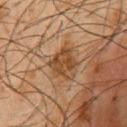Assessment:
The lesion was tiled from a total-body skin photograph and was not biopsied.
Image and clinical context:
Imaged with cross-polarized lighting. Approximately 4 mm at its widest. Automated image analysis of the tile measured a normalized lesion–skin contrast near 8. A 15 mm close-up tile from a total-body photography series done for melanoma screening. The lesion is located on the front of the torso. A male subject, roughly 60 years of age.